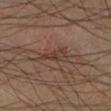notes: total-body-photography surveillance lesion; no biopsy
subject: male, about 40 years old
lesion diameter: ≈3.5 mm
anatomic site: the left lower leg
image: ~15 mm tile from a whole-body skin photo
lighting: cross-polarized illumination